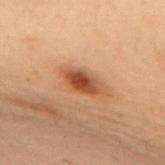Case summary:
* biopsy status: imaged on a skin check; not biopsied
* imaging modality: ~15 mm crop, total-body skin-cancer survey
* tile lighting: cross-polarized
* body site: the upper back
* subject: female, in their mid- to late 50s
* automated metrics: a lesion area of about 7.5 mm², an outline eccentricity of about 0.9 (0 = round, 1 = elongated), and a shape-asymmetry score of about 0.15 (0 = symmetric); an average lesion color of about L≈42 a*≈23 b*≈32 (CIELAB), about 12 CIELAB-L* units darker than the surrounding skin, and a normalized lesion–skin contrast near 9.5
* lesion diameter: ≈4.5 mm